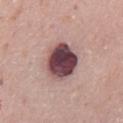Clinical impression:
Recorded during total-body skin imaging; not selected for excision or biopsy.
Acquisition and patient details:
Measured at roughly 5 mm in maximum diameter. A 15 mm close-up tile from a total-body photography series done for melanoma screening. The patient is a male roughly 70 years of age. The lesion is located on the abdomen. The tile uses white-light illumination. An algorithmic analysis of the crop reported a lesion area of about 15 mm², an eccentricity of roughly 0.6, and two-axis asymmetry of about 0.1. It also reported a lesion color around L≈44 a*≈22 b*≈15 in CIELAB, about 23 CIELAB-L* units darker than the surrounding skin, and a normalized border contrast of about 16. And it measured a lesion-detection confidence of about 100/100.